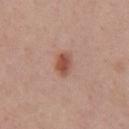Image and clinical context:
Automated image analysis of the tile measured an area of roughly 5 mm², an eccentricity of roughly 0.75, and a symmetry-axis asymmetry near 0.15. It also reported a lesion color around L≈52 a*≈24 b*≈29 in CIELAB and a normalized border contrast of about 8.5. And it measured a border-irregularity index near 1.5/10, internal color variation of about 4.5 on a 0–10 scale, and peripheral color asymmetry of about 1.5. A male patient aged around 60. On the front of the torso. A lesion tile, about 15 mm wide, cut from a 3D total-body photograph. Captured under white-light illumination. The lesion's longest dimension is about 3 mm.